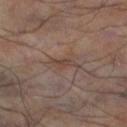Q: Was this lesion biopsied?
A: imaged on a skin check; not biopsied
Q: What did automated image analysis measure?
A: a footprint of about 3 mm², a shape eccentricity near 0.85, and a shape-asymmetry score of about 0.45 (0 = symmetric); a lesion color around L≈39 a*≈15 b*≈22 in CIELAB, a lesion–skin lightness drop of about 6, and a normalized lesion–skin contrast near 6; an automated nevus-likeness rating near 0 out of 100 and a detector confidence of about 95 out of 100 that the crop contains a lesion
Q: Where on the body is the lesion?
A: the left lower leg
Q: How was this image acquired?
A: ~15 mm crop, total-body skin-cancer survey
Q: Who is the patient?
A: male, about 55 years old
Q: Illumination type?
A: cross-polarized illumination
Q: Lesion size?
A: ~2.5 mm (longest diameter)